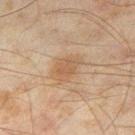| key | value |
|---|---|
| biopsy status | catalogued during a skin exam; not biopsied |
| lighting | cross-polarized |
| subject | male, aged around 45 |
| acquisition | 15 mm crop, total-body photography |
| lesion diameter | about 3.5 mm |
| automated lesion analysis | a footprint of about 6 mm² and an eccentricity of roughly 0.75; border irregularity of about 3 on a 0–10 scale |
| anatomic site | the right thigh |